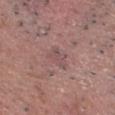Assessment: Recorded during total-body skin imaging; not selected for excision or biopsy. Background: The recorded lesion diameter is about 3.5 mm. Imaged with white-light lighting. A male subject roughly 70 years of age. The lesion is located on the chest. Automated tile analysis of the lesion measured a lesion color around L≈50 a*≈20 b*≈19 in CIELAB, a lesion–skin lightness drop of about 6, and a lesion-to-skin contrast of about 5 (normalized; higher = more distinct). Cropped from a whole-body photographic skin survey; the tile spans about 15 mm.